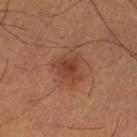Q: Was a biopsy performed?
A: catalogued during a skin exam; not biopsied
Q: Lesion location?
A: the left thigh
Q: What is the lesion's diameter?
A: ~3 mm (longest diameter)
Q: Illumination type?
A: cross-polarized illumination
Q: Patient demographics?
A: male, aged 48 to 52
Q: Automated lesion metrics?
A: an area of roughly 6.5 mm², a shape eccentricity near 0.6, and a shape-asymmetry score of about 0.25 (0 = symmetric); a mean CIELAB color near L≈41 a*≈26 b*≈31, roughly 8 lightness units darker than nearby skin, and a normalized lesion–skin contrast near 6.5; a border-irregularity rating of about 2.5/10 and a color-variation rating of about 3/10
Q: What is the imaging modality?
A: ~15 mm tile from a whole-body skin photo